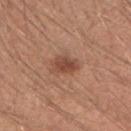The lesion was photographed on a routine skin check and not biopsied; there is no pathology result. Automated image analysis of the tile measured roughly 11 lightness units darker than nearby skin and a normalized border contrast of about 8. It also reported a within-lesion color-variation index near 3/10 and radial color variation of about 1. Longest diameter approximately 3 mm. This is a white-light tile. The lesion is on the arm. The subject is a male aged approximately 30. A 15 mm crop from a total-body photograph taken for skin-cancer surveillance.